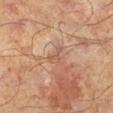notes: total-body-photography surveillance lesion; no biopsy
patient: male, about 65 years old
imaging modality: 15 mm crop, total-body photography
location: the leg